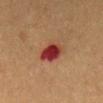Image and clinical context:
An algorithmic analysis of the crop reported a mean CIELAB color near L≈31 a*≈27 b*≈24, roughly 13 lightness units darker than nearby skin, and a lesion-to-skin contrast of about 12 (normalized; higher = more distinct). And it measured an automated nevus-likeness rating near 0 out of 100. This is a cross-polarized tile. A male patient, about 50 years old. Longest diameter approximately 3.5 mm. A 15 mm crop from a total-body photograph taken for skin-cancer surveillance. Located on the mid back.